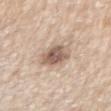Assessment: The lesion was photographed on a routine skin check and not biopsied; there is no pathology result. Context: The recorded lesion diameter is about 4 mm. A male subject in their mid-70s. This image is a 15 mm lesion crop taken from a total-body photograph. On the right upper arm. The tile uses white-light illumination.